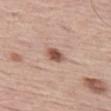Impression: Imaged during a routine full-body skin examination; the lesion was not biopsied and no histopathology is available. Acquisition and patient details: A 15 mm close-up tile from a total-body photography series done for melanoma screening. The subject is a male in their mid-70s. Automated image analysis of the tile measured an area of roughly 4 mm² and an eccentricity of roughly 0.7. The analysis additionally found a lesion color around L≈53 a*≈22 b*≈27 in CIELAB, a lesion–skin lightness drop of about 14, and a normalized lesion–skin contrast near 9.5. It also reported a border-irregularity rating of about 2/10 and a within-lesion color-variation index near 3.5/10. The analysis additionally found a classifier nevus-likeness of about 95/100. The lesion is on the left thigh.